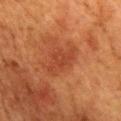A female subject, aged 53–57.
The recorded lesion diameter is about 4.5 mm.
From the mid back.
The tile uses cross-polarized illumination.
A lesion tile, about 15 mm wide, cut from a 3D total-body photograph.